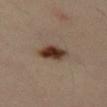The lesion was photographed on a routine skin check and not biopsied; there is no pathology result.
Automated tile analysis of the lesion measured a footprint of about 8 mm² and a symmetry-axis asymmetry near 0.15. And it measured border irregularity of about 2 on a 0–10 scale, a color-variation rating of about 6/10, and peripheral color asymmetry of about 2. The analysis additionally found a nevus-likeness score of about 100/100.
A close-up tile cropped from a whole-body skin photograph, about 15 mm across.
The lesion is located on the left thigh.
The patient is a male in their 40s.
The tile uses cross-polarized illumination.
The recorded lesion diameter is about 4 mm.